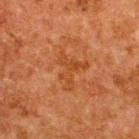No biopsy was performed on this lesion — it was imaged during a full skin examination and was not determined to be concerning. The recorded lesion diameter is about 4.5 mm. This image is a 15 mm lesion crop taken from a total-body photograph. The tile uses cross-polarized illumination. Automated tile analysis of the lesion measured an average lesion color of about L≈36 a*≈23 b*≈34 (CIELAB). And it measured a classifier nevus-likeness of about 0/100 and a lesion-detection confidence of about 100/100. The lesion is located on the upper back. A male patient approximately 60 years of age.